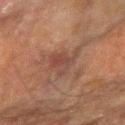biopsy status: catalogued during a skin exam; not biopsied | diameter: about 4 mm | automated lesion analysis: a mean CIELAB color near L≈35 a*≈17 b*≈22, about 5 CIELAB-L* units darker than the surrounding skin, and a normalized border contrast of about 5.5 | imaging modality: total-body-photography crop, ~15 mm field of view | patient: male, aged 63–67 | body site: the left forearm | illumination: cross-polarized illumination.